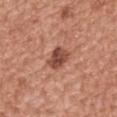Context: Imaged with white-light lighting. A lesion tile, about 15 mm wide, cut from a 3D total-body photograph. The lesion is on the chest. The patient is a male roughly 45 years of age. The lesion-visualizer software estimated a lesion area of about 5.5 mm² and a shape eccentricity near 0.5. Measured at roughly 2.5 mm in maximum diameter.